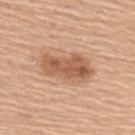Findings:
- biopsy status — catalogued during a skin exam; not biopsied
- image — total-body-photography crop, ~15 mm field of view
- subject — female, aged 58 to 62
- anatomic site — the back